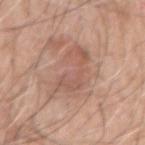Part of a total-body skin-imaging series; this lesion was reviewed on a skin check and was not flagged for biopsy.
The patient is a male aged 58 to 62.
On the arm.
A 15 mm close-up extracted from a 3D total-body photography capture.
An algorithmic analysis of the crop reported a shape eccentricity near 0.9 and two-axis asymmetry of about 0.4. It also reported a border-irregularity index near 7.5/10, internal color variation of about 3.5 on a 0–10 scale, and a peripheral color-asymmetry measure near 1.
Approximately 7 mm at its widest.